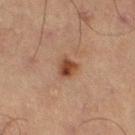{
  "biopsy_status": "not biopsied; imaged during a skin examination",
  "site": "left thigh",
  "automated_metrics": {
    "vs_skin_darker_L": 10.0,
    "vs_skin_contrast_norm": 9.5,
    "peripheral_color_asymmetry": 1.5,
    "nevus_likeness_0_100": 95
  },
  "lighting": "cross-polarized",
  "patient": {
    "sex": "male",
    "age_approx": 65
  },
  "image": {
    "source": "total-body photography crop",
    "field_of_view_mm": 15
  }
}